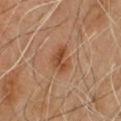No biopsy was performed on this lesion — it was imaged during a full skin examination and was not determined to be concerning.
The lesion's longest dimension is about 3 mm.
The subject is a male about 55 years old.
Imaged with cross-polarized lighting.
A roughly 15 mm field-of-view crop from a total-body skin photograph.
The lesion is located on the chest.